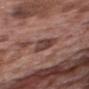The lesion was photographed on a routine skin check and not biopsied; there is no pathology result.